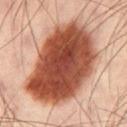Q: Is there a histopathology result?
A: imaged on a skin check; not biopsied
Q: What kind of image is this?
A: 15 mm crop, total-body photography
Q: Where on the body is the lesion?
A: the leg
Q: Illumination type?
A: cross-polarized
Q: Lesion size?
A: ~11.5 mm (longest diameter)
Q: What did automated image analysis measure?
A: a classifier nevus-likeness of about 100/100 and lesion-presence confidence of about 100/100
Q: Who is the patient?
A: male, in their 40s